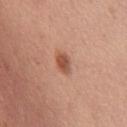{
  "biopsy_status": "not biopsied; imaged during a skin examination",
  "site": "chest",
  "patient": {
    "sex": "female",
    "age_approx": 45
  },
  "image": {
    "source": "total-body photography crop",
    "field_of_view_mm": 15
  },
  "lighting": "white-light",
  "lesion_size": {
    "long_diameter_mm_approx": 2.5
  }
}